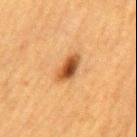tile lighting = cross-polarized illumination
automated lesion analysis = an automated nevus-likeness rating near 100 out of 100 and lesion-presence confidence of about 100/100
size = ≈4 mm
image source = ~15 mm tile from a whole-body skin photo
patient = female, about 55 years old
site = the mid back